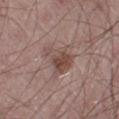Impression: Recorded during total-body skin imaging; not selected for excision or biopsy. Background: Imaged with white-light lighting. Located on the left thigh. A roughly 15 mm field-of-view crop from a total-body skin photograph. The recorded lesion diameter is about 5 mm. A male patient, aged around 65.